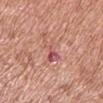Impression:
Captured during whole-body skin photography for melanoma surveillance; the lesion was not biopsied.
Background:
A 15 mm close-up tile from a total-body photography series done for melanoma screening. Approximately 4 mm at its widest. The patient is a male aged approximately 65. Located on the left upper arm. The tile uses white-light illumination.